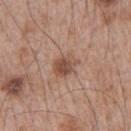This lesion was catalogued during total-body skin photography and was not selected for biopsy. A male patient, aged 63–67. Captured under white-light illumination. A roughly 15 mm field-of-view crop from a total-body skin photograph. Approximately 3 mm at its widest. The lesion is on the left upper arm.